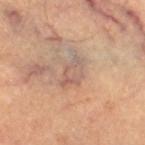biopsy status: imaged on a skin check; not biopsied | body site: the right thigh | lighting: cross-polarized illumination | image-analysis metrics: an eccentricity of roughly 0.85; an average lesion color of about L≈56 a*≈17 b*≈25 (CIELAB), a lesion–skin lightness drop of about 7, and a lesion-to-skin contrast of about 5.5 (normalized; higher = more distinct); a nevus-likeness score of about 0/100 and a detector confidence of about 70 out of 100 that the crop contains a lesion | patient: male, roughly 60 years of age | imaging modality: ~15 mm crop, total-body skin-cancer survey | diameter: ~4 mm (longest diameter).